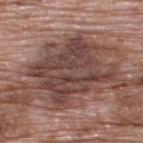workup: catalogued during a skin exam; not biopsied
image source: ~15 mm crop, total-body skin-cancer survey
patient: male, in their 70s
site: the upper back